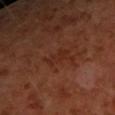The lesion was tiled from a total-body skin photograph and was not biopsied.
Automated image analysis of the tile measured a footprint of about 4 mm² and an eccentricity of roughly 0.9. The analysis additionally found a lesion color around L≈28 a*≈24 b*≈29 in CIELAB and a normalized lesion–skin contrast near 5. And it measured a nevus-likeness score of about 0/100 and lesion-presence confidence of about 100/100.
A lesion tile, about 15 mm wide, cut from a 3D total-body photograph.
A female patient, approximately 50 years of age.
Imaged with cross-polarized lighting.
Approximately 3 mm at its widest.
From the left forearm.